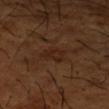Q: Was a biopsy performed?
A: imaged on a skin check; not biopsied
Q: What is the anatomic site?
A: the right forearm
Q: What lighting was used for the tile?
A: cross-polarized illumination
Q: Patient demographics?
A: male, aged approximately 65
Q: How was this image acquired?
A: total-body-photography crop, ~15 mm field of view